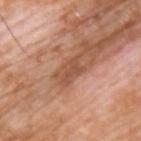Recorded during total-body skin imaging; not selected for excision or biopsy. The lesion is on the upper back. A male subject, roughly 60 years of age. Measured at roughly 3.5 mm in maximum diameter. The lesion-visualizer software estimated a normalized border contrast of about 6.5. It also reported a detector confidence of about 100 out of 100 that the crop contains a lesion. This image is a 15 mm lesion crop taken from a total-body photograph. This is a white-light tile.